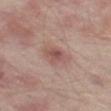Notes:
– image · ~15 mm tile from a whole-body skin photo
– body site · the leg
– diameter · about 3 mm
– patient · male, roughly 65 years of age